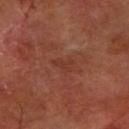Part of a total-body skin-imaging series; this lesion was reviewed on a skin check and was not flagged for biopsy. Imaged with cross-polarized lighting. A 15 mm close-up extracted from a 3D total-body photography capture. A patient aged 63–67. Located on the right forearm. Longest diameter approximately 2.5 mm.